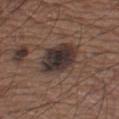– biopsy status · catalogued during a skin exam; not biopsied
– anatomic site · the left thigh
– patient · male, aged approximately 65
– automated lesion analysis · roughly 12 lightness units darker than nearby skin; a classifier nevus-likeness of about 25/100 and a lesion-detection confidence of about 100/100
– lesion diameter · ~6 mm (longest diameter)
– acquisition · 15 mm crop, total-body photography